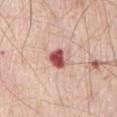- workup — no biopsy performed (imaged during a skin exam)
- site — the abdomen
- lighting — white-light
- patient — male, aged around 80
- image source — total-body-photography crop, ~15 mm field of view